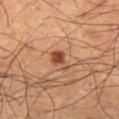Q: Was this lesion biopsied?
A: catalogued during a skin exam; not biopsied
Q: How was the tile lit?
A: cross-polarized
Q: What is the anatomic site?
A: the left thigh
Q: What is the imaging modality?
A: ~15 mm tile from a whole-body skin photo
Q: What are the patient's age and sex?
A: male, aged approximately 60
Q: Lesion size?
A: ≈3 mm